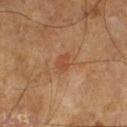notes: no biopsy performed (imaged during a skin exam) | automated lesion analysis: an area of roughly 3.5 mm², a shape eccentricity near 0.75, and a symmetry-axis asymmetry near 0.2; a mean CIELAB color near L≈46 a*≈23 b*≈34, about 6 CIELAB-L* units darker than the surrounding skin, and a normalized lesion–skin contrast near 5; a lesion-detection confidence of about 100/100 | anatomic site: the left lower leg | diameter: ≈2.5 mm | lighting: cross-polarized illumination | image source: ~15 mm tile from a whole-body skin photo | subject: male, approximately 65 years of age.